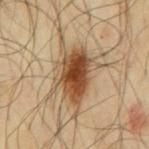Part of a total-body skin-imaging series; this lesion was reviewed on a skin check and was not flagged for biopsy.
The patient is a male aged around 65.
Located on the mid back.
Approximately 8.5 mm at its widest.
Imaged with cross-polarized lighting.
A 15 mm close-up tile from a total-body photography series done for melanoma screening.